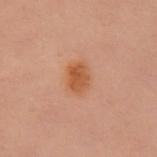Imaged during a routine full-body skin examination; the lesion was not biopsied and no histopathology is available. Cropped from a total-body skin-imaging series; the visible field is about 15 mm. The lesion is on the chest. The tile uses cross-polarized illumination. A female patient, in their 60s. The recorded lesion diameter is about 3.5 mm.